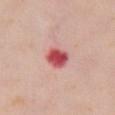Imaged during a routine full-body skin examination; the lesion was not biopsied and no histopathology is available. From the chest. The tile uses white-light illumination. Longest diameter approximately 3 mm. A female subject approximately 60 years of age. A roughly 15 mm field-of-view crop from a total-body skin photograph.